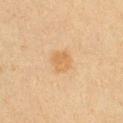Assessment:
The lesion was photographed on a routine skin check and not biopsied; there is no pathology result.
Background:
Located on the chest. A 15 mm close-up extracted from a 3D total-body photography capture. The patient is a female in their 20s. Approximately 3 mm at its widest.